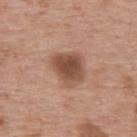Impression: This lesion was catalogued during total-body skin photography and was not selected for biopsy. Image and clinical context: Imaged with white-light lighting. A roughly 15 mm field-of-view crop from a total-body skin photograph. The lesion is on the upper back. The patient is a male aged 73 to 77.